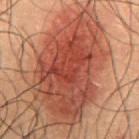Q: Is there a histopathology result?
A: no biopsy performed (imaged during a skin exam)
Q: Patient demographics?
A: male, aged 48 to 52
Q: How was the tile lit?
A: cross-polarized
Q: Lesion size?
A: ~12.5 mm (longest diameter)
Q: What did automated image analysis measure?
A: an area of roughly 60 mm², an outline eccentricity of about 0.8 (0 = round, 1 = elongated), and a shape-asymmetry score of about 0.25 (0 = symmetric)
Q: What is the imaging modality?
A: ~15 mm tile from a whole-body skin photo
Q: Where on the body is the lesion?
A: the abdomen